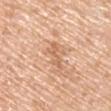Background: The lesion is on the arm. A lesion tile, about 15 mm wide, cut from a 3D total-body photograph. Longest diameter approximately 4 mm. A male patient aged 68 to 72. Imaged with white-light lighting.